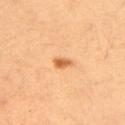biopsy_status: not biopsied; imaged during a skin examination
image:
  source: total-body photography crop
  field_of_view_mm: 15
site: left upper arm
patient:
  sex: female
  age_approx: 35